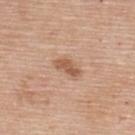– notes — catalogued during a skin exam; not biopsied
– image source — ~15 mm crop, total-body skin-cancer survey
– patient — female, aged around 65
– automated metrics — a lesion area of about 4 mm², a shape eccentricity near 0.9, and a symmetry-axis asymmetry near 0.35; an average lesion color of about L≈56 a*≈21 b*≈32 (CIELAB), roughly 11 lightness units darker than nearby skin, and a normalized border contrast of about 8; border irregularity of about 3.5 on a 0–10 scale, internal color variation of about 1 on a 0–10 scale, and peripheral color asymmetry of about 0.5
– anatomic site — the upper back
– illumination — white-light
– size — ~3 mm (longest diameter)